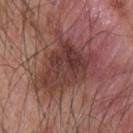| field | value |
|---|---|
| biopsy status | total-body-photography surveillance lesion; no biopsy |
| anatomic site | the upper back |
| illumination | white-light illumination |
| acquisition | ~15 mm crop, total-body skin-cancer survey |
| subject | male, in their mid- to late 40s |
| lesion diameter | ≈7.5 mm |